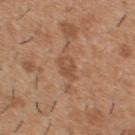Q: Is there a histopathology result?
A: catalogued during a skin exam; not biopsied
Q: Lesion size?
A: ≈3 mm
Q: Lesion location?
A: the upper back
Q: Automated lesion metrics?
A: a lesion area of about 4.5 mm² and a symmetry-axis asymmetry near 0.25; a border-irregularity index near 2.5/10
Q: Who is the patient?
A: male, aged around 40
Q: What is the imaging modality?
A: ~15 mm tile from a whole-body skin photo
Q: What lighting was used for the tile?
A: white-light illumination